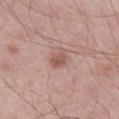{
  "biopsy_status": "not biopsied; imaged during a skin examination",
  "patient": {
    "sex": "male",
    "age_approx": 55
  },
  "image": {
    "source": "total-body photography crop",
    "field_of_view_mm": 15
  },
  "lesion_size": {
    "long_diameter_mm_approx": 3.0
  },
  "automated_metrics": {
    "eccentricity": 0.8,
    "shape_asymmetry": 0.25,
    "color_variation_0_10": 2.0,
    "peripheral_color_asymmetry": 0.5
  },
  "lighting": "white-light",
  "site": "leg"
}